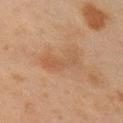{"biopsy_status": "not biopsied; imaged during a skin examination", "lighting": "cross-polarized", "lesion_size": {"long_diameter_mm_approx": 5.0}, "image": {"source": "total-body photography crop", "field_of_view_mm": 15}, "patient": {"sex": "male", "age_approx": 45}, "site": "right upper arm"}